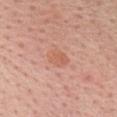image: ~15 mm crop, total-body skin-cancer survey | automated lesion analysis: a lesion area of about 5.5 mm²; a mean CIELAB color near L≈61 a*≈25 b*≈32, roughly 6 lightness units darker than nearby skin, and a normalized border contrast of about 5; a classifier nevus-likeness of about 10/100 and a detector confidence of about 100 out of 100 that the crop contains a lesion | size: ~3 mm (longest diameter) | lighting: white-light illumination | body site: the chest | patient: female, aged 43 to 47.